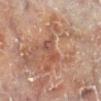Acquisition and patient details: A lesion tile, about 15 mm wide, cut from a 3D total-body photograph. Captured under cross-polarized illumination. The patient is a male aged 58–62. On the left lower leg. Approximately 3 mm at its widest.